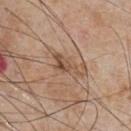<lesion>
<biopsy_status>not biopsied; imaged during a skin examination</biopsy_status>
<site>chest</site>
<patient>
  <sex>male</sex>
  <age_approx>65</age_approx>
</patient>
<image>
  <source>total-body photography crop</source>
  <field_of_view_mm>15</field_of_view_mm>
</image>
</lesion>